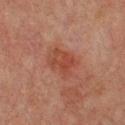follow-up: imaged on a skin check; not biopsied | tile lighting: cross-polarized illumination | patient: male, in their mid- to late 60s | site: the back | image: total-body-photography crop, ~15 mm field of view | image-analysis metrics: a border-irregularity rating of about 2.5/10 | lesion size: about 4 mm.